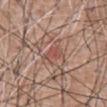notes — total-body-photography surveillance lesion; no biopsy
size — about 3 mm
patient — male, aged 58–62
site — the front of the torso
tile lighting — white-light
imaging modality — total-body-photography crop, ~15 mm field of view